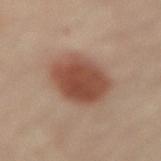Recorded during total-body skin imaging; not selected for excision or biopsy. A female subject, about 60 years old. On the lower back. A 15 mm crop from a total-body photograph taken for skin-cancer surveillance.